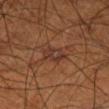Image and clinical context: The lesion is on the right lower leg. Automated image analysis of the tile measured a lesion area of about 5 mm² and two-axis asymmetry of about 0.15. The software also gave a mean CIELAB color near L≈34 a*≈20 b*≈26, a lesion–skin lightness drop of about 6, and a lesion-to-skin contrast of about 7 (normalized; higher = more distinct). And it measured a border-irregularity index near 1.5/10, internal color variation of about 4 on a 0–10 scale, and peripheral color asymmetry of about 1.5. The analysis additionally found an automated nevus-likeness rating near 0 out of 100. A male patient aged 68–72. A 15 mm close-up extracted from a 3D total-body photography capture. The tile uses cross-polarized illumination.